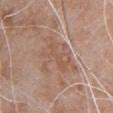biopsy status: total-body-photography surveillance lesion; no biopsy | acquisition: 15 mm crop, total-body photography | lesion diameter: ≈5.5 mm | TBP lesion metrics: a normalized lesion–skin contrast near 5 | tile lighting: white-light | anatomic site: the front of the torso | patient: male, aged 78–82.